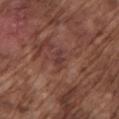Recorded during total-body skin imaging; not selected for excision or biopsy.
A lesion tile, about 15 mm wide, cut from a 3D total-body photograph.
This is a white-light tile.
The patient is a male aged around 75.
Automated tile analysis of the lesion measured a footprint of about 3 mm², an eccentricity of roughly 0.85, and a shape-asymmetry score of about 0.2 (0 = symmetric). It also reported a lesion color around L≈37 a*≈22 b*≈21 in CIELAB and a lesion-to-skin contrast of about 6.5 (normalized; higher = more distinct).
From the left upper arm.